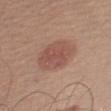workup = catalogued during a skin exam; not biopsied | lesion size = ≈5.5 mm | lighting = white-light illumination | patient = male, aged 53 to 57 | acquisition = ~15 mm crop, total-body skin-cancer survey | body site = the left upper arm.